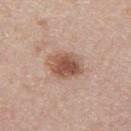Impression:
Imaged during a routine full-body skin examination; the lesion was not biopsied and no histopathology is available.
Clinical summary:
A female patient, about 55 years old. Located on the upper back. This is a white-light tile. A lesion tile, about 15 mm wide, cut from a 3D total-body photograph.